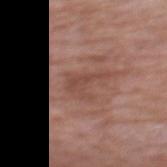Notes:
* follow-up · total-body-photography surveillance lesion; no biopsy
* patient · female, roughly 60 years of age
* anatomic site · the upper back
* acquisition · ~15 mm tile from a whole-body skin photo
* size · about 4.5 mm
* illumination · white-light illumination